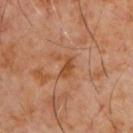Impression:
This lesion was catalogued during total-body skin photography and was not selected for biopsy.
Image and clinical context:
From the front of the torso. Automated image analysis of the tile measured a classifier nevus-likeness of about 0/100. A male patient, aged 58–62. A roughly 15 mm field-of-view crop from a total-body skin photograph.